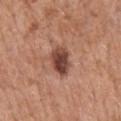Recorded during total-body skin imaging; not selected for excision or biopsy. Captured under white-light illumination. From the back. The total-body-photography lesion software estimated a border-irregularity rating of about 2.5/10, a within-lesion color-variation index near 6/10, and radial color variation of about 2. The software also gave lesion-presence confidence of about 100/100. Measured at roughly 3.5 mm in maximum diameter. A lesion tile, about 15 mm wide, cut from a 3D total-body photograph. A male patient aged approximately 70.